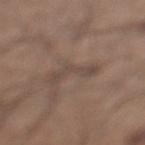notes = catalogued during a skin exam; not biopsied | subject = male, aged around 60 | site = the left lower leg | imaging modality = 15 mm crop, total-body photography | size = ≈4 mm | TBP lesion metrics = a lesion area of about 5 mm², a shape eccentricity near 0.85, and a symmetry-axis asymmetry near 0.75; a mean CIELAB color near L≈45 a*≈14 b*≈22 and about 6 CIELAB-L* units darker than the surrounding skin; a classifier nevus-likeness of about 0/100 and a detector confidence of about 90 out of 100 that the crop contains a lesion.